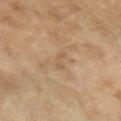A 15 mm close-up tile from a total-body photography series done for melanoma screening. Located on the arm. The subject is a female aged around 60. The tile uses cross-polarized illumination.